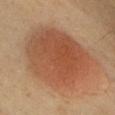{
  "biopsy_status": "not biopsied; imaged during a skin examination",
  "site": "chest",
  "lesion_size": {
    "long_diameter_mm_approx": 8.5
  },
  "patient": {
    "sex": "male",
    "age_approx": 40
  },
  "image": {
    "source": "total-body photography crop",
    "field_of_view_mm": 15
  }
}